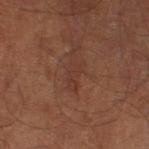<lesion>
  <biopsy_status>not biopsied; imaged during a skin examination</biopsy_status>
  <automated_metrics>
    <cielab_L>32</cielab_L>
    <cielab_a>19</cielab_a>
    <cielab_b>24</cielab_b>
    <vs_skin_darker_L>5.0</vs_skin_darker_L>
    <vs_skin_contrast_norm>5.0</vs_skin_contrast_norm>
    <nevus_likeness_0_100>0</nevus_likeness_0_100>
    <lesion_detection_confidence_0_100>90</lesion_detection_confidence_0_100>
  </automated_metrics>
  <patient>
    <sex>male</sex>
    <age_approx>60</age_approx>
  </patient>
  <site>left lower leg</site>
  <lighting>cross-polarized</lighting>
  <lesion_size>
    <long_diameter_mm_approx>3.5</long_diameter_mm_approx>
  </lesion_size>
  <image>
    <source>total-body photography crop</source>
    <field_of_view_mm>15</field_of_view_mm>
  </image>
</lesion>